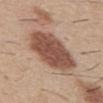This lesion was catalogued during total-body skin photography and was not selected for biopsy. Located on the abdomen. A close-up tile cropped from a whole-body skin photograph, about 15 mm across. Measured at roughly 8 mm in maximum diameter. A male subject roughly 30 years of age. The tile uses white-light illumination.